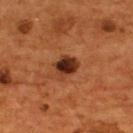Q: Is there a histopathology result?
A: catalogued during a skin exam; not biopsied
Q: How was the tile lit?
A: cross-polarized
Q: What kind of image is this?
A: total-body-photography crop, ~15 mm field of view
Q: Lesion size?
A: ~3 mm (longest diameter)
Q: Patient demographics?
A: male, aged 53 to 57
Q: Where on the body is the lesion?
A: the upper back